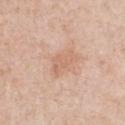Notes:
- workup · total-body-photography surveillance lesion; no biopsy
- automated lesion analysis · a footprint of about 5.5 mm², an outline eccentricity of about 0.85 (0 = round, 1 = elongated), and a symmetry-axis asymmetry near 0.4; an average lesion color of about L≈65 a*≈20 b*≈31 (CIELAB), a lesion–skin lightness drop of about 7, and a normalized lesion–skin contrast near 5; a nevus-likeness score of about 0/100 and a detector confidence of about 100 out of 100 that the crop contains a lesion
- tile lighting · white-light illumination
- lesion size · about 3.5 mm
- site · the chest
- image · ~15 mm tile from a whole-body skin photo
- patient · female, aged approximately 40